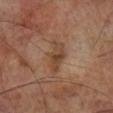  biopsy_status: not biopsied; imaged during a skin examination
  image:
    source: total-body photography crop
    field_of_view_mm: 15
  patient:
    sex: male
    age_approx: 70
  site: leg